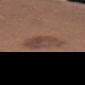{
  "biopsy_status": "not biopsied; imaged during a skin examination"
}